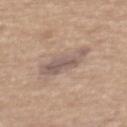biopsy status: no biopsy performed (imaged during a skin exam) | lesion diameter: ~5 mm (longest diameter) | image source: total-body-photography crop, ~15 mm field of view | location: the mid back | subject: male, aged around 65 | illumination: white-light.